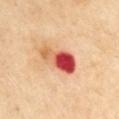Clinical summary: This image is a 15 mm lesion crop taken from a total-body photograph. The lesion is located on the left upper arm. A male patient, roughly 70 years of age.